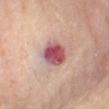<tbp_lesion>
  <biopsy_status>not biopsied; imaged during a skin examination</biopsy_status>
  <site>left thigh</site>
  <patient>
    <sex>female</sex>
    <age_approx>60</age_approx>
  </patient>
  <image>
    <source>total-body photography crop</source>
    <field_of_view_mm>15</field_of_view_mm>
  </image>
  <lighting>cross-polarized</lighting>
</tbp_lesion>